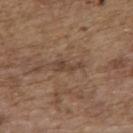workup: total-body-photography surveillance lesion; no biopsy | imaging modality: total-body-photography crop, ~15 mm field of view | body site: the upper back | tile lighting: white-light illumination | subject: female, aged 63 to 67.